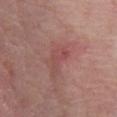Impression:
Captured during whole-body skin photography for melanoma surveillance; the lesion was not biopsied.
Acquisition and patient details:
The lesion is located on the chest. A male subject aged 78–82. A 15 mm close-up tile from a total-body photography series done for melanoma screening. The recorded lesion diameter is about 3 mm. The tile uses white-light illumination.